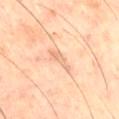notes: no biopsy performed (imaged during a skin exam) | subject: male, in their 60s | TBP lesion metrics: an area of roughly 3.5 mm², an eccentricity of roughly 0.9, and a shape-asymmetry score of about 0.4 (0 = symmetric); a lesion color around L≈70 a*≈21 b*≈34 in CIELAB, roughly 8 lightness units darker than nearby skin, and a normalized lesion–skin contrast near 5 | location: the right thigh | image: 15 mm crop, total-body photography | lighting: cross-polarized.